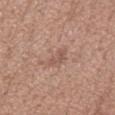<lesion>
  <patient>
    <sex>female</sex>
    <age_approx>30</age_approx>
  </patient>
  <lesion_size>
    <long_diameter_mm_approx>2.5</long_diameter_mm_approx>
  </lesion_size>
  <lighting>white-light</lighting>
  <image>
    <source>total-body photography crop</source>
    <field_of_view_mm>15</field_of_view_mm>
  </image>
  <site>left forearm</site>
</lesion>